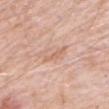workup: catalogued during a skin exam; not biopsied
lighting: white-light
anatomic site: the abdomen
TBP lesion metrics: a lesion color around L≈64 a*≈21 b*≈31 in CIELAB, roughly 8 lightness units darker than nearby skin, and a lesion-to-skin contrast of about 5.5 (normalized; higher = more distinct); a border-irregularity rating of about 6.5/10, a color-variation rating of about 0/10, and a peripheral color-asymmetry measure near 0; a detector confidence of about 85 out of 100 that the crop contains a lesion
diameter: ≈3 mm
acquisition: ~15 mm crop, total-body skin-cancer survey
subject: male, about 80 years old